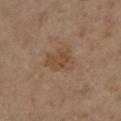Background:
An algorithmic analysis of the crop reported a lesion area of about 8 mm², a shape eccentricity near 0.7, and a shape-asymmetry score of about 0.3 (0 = symmetric). The analysis additionally found a lesion color around L≈46 a*≈17 b*≈30 in CIELAB, roughly 7 lightness units darker than nearby skin, and a normalized lesion–skin contrast near 6. The software also gave a nevus-likeness score of about 5/100. About 4 mm across. A roughly 15 mm field-of-view crop from a total-body skin photograph. A male subject roughly 65 years of age. From the leg.